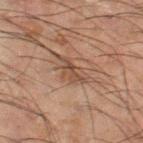notes = imaged on a skin check; not biopsied | patient = male, about 65 years old | anatomic site = the leg | acquisition = 15 mm crop, total-body photography | illumination = cross-polarized illumination | lesion size = ~5 mm (longest diameter) | automated lesion analysis = border irregularity of about 5.5 on a 0–10 scale, internal color variation of about 4 on a 0–10 scale, and peripheral color asymmetry of about 1.5.